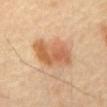The lesion was tiled from a total-body skin photograph and was not biopsied.
A male patient, about 45 years old.
This image is a 15 mm lesion crop taken from a total-body photograph.
From the front of the torso.